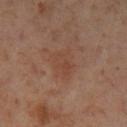Impression:
This lesion was catalogued during total-body skin photography and was not selected for biopsy.
Image and clinical context:
The lesion is on the left lower leg. The recorded lesion diameter is about 3.5 mm. A female subject aged 53 to 57. Imaged with cross-polarized lighting. The total-body-photography lesion software estimated a lesion area of about 8.5 mm² and an outline eccentricity of about 0.7 (0 = round, 1 = elongated). It also reported a lesion color around L≈45 a*≈20 b*≈29 in CIELAB, about 5 CIELAB-L* units darker than the surrounding skin, and a normalized lesion–skin contrast near 4.5. The software also gave a peripheral color-asymmetry measure near 1. A 15 mm close-up tile from a total-body photography series done for melanoma screening.